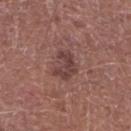Recorded during total-body skin imaging; not selected for excision or biopsy. The subject is a male aged around 75. This is a white-light tile. Approximately 3.5 mm at its widest. The lesion is on the leg. A region of skin cropped from a whole-body photographic capture, roughly 15 mm wide.